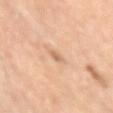Imaged during a routine full-body skin examination; the lesion was not biopsied and no histopathology is available.
A female subject aged around 65.
A 15 mm close-up extracted from a 3D total-body photography capture.
Located on the right upper arm.